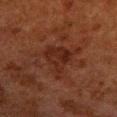<tbp_lesion>
  <biopsy_status>not biopsied; imaged during a skin examination</biopsy_status>
  <image>
    <source>total-body photography crop</source>
    <field_of_view_mm>15</field_of_view_mm>
  </image>
  <site>leg</site>
  <automated_metrics>
    <area_mm2_approx>10.0</area_mm2_approx>
    <eccentricity>0.6</eccentricity>
    <shape_asymmetry>0.55</shape_asymmetry>
    <color_variation_0_10>3.0</color_variation_0_10>
    <peripheral_color_asymmetry>1.0</peripheral_color_asymmetry>
  </automated_metrics>
  <lesion_size>
    <long_diameter_mm_approx>5.0</long_diameter_mm_approx>
  </lesion_size>
  <patient>
    <sex>male</sex>
    <age_approx>80</age_approx>
  </patient>
</tbp_lesion>